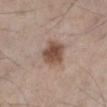biopsy_status: not biopsied; imaged during a skin examination
automated_metrics:
  area_mm2_approx: 9.5
  cielab_L: 49
  cielab_a: 17
  cielab_b: 26
  vs_skin_darker_L: 13.0
  border_irregularity_0_10: 2.0
  nevus_likeness_0_100: 95
lesion_size:
  long_diameter_mm_approx: 3.5
site: left lower leg
lighting: white-light
patient:
  sex: male
  age_approx: 60
image:
  source: total-body photography crop
  field_of_view_mm: 15